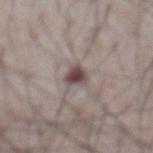Part of a total-body skin-imaging series; this lesion was reviewed on a skin check and was not flagged for biopsy.
A 15 mm close-up extracted from a 3D total-body photography capture.
A male subject roughly 75 years of age.
Located on the abdomen.
Captured under white-light illumination.
An algorithmic analysis of the crop reported a footprint of about 5 mm², a shape eccentricity near 0.65, and a symmetry-axis asymmetry near 0.35. The analysis additionally found a lesion color around L≈47 a*≈15 b*≈14 in CIELAB, a lesion–skin lightness drop of about 14, and a normalized border contrast of about 10.5.